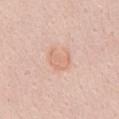Recorded during total-body skin imaging; not selected for excision or biopsy.
Measured at roughly 3.5 mm in maximum diameter.
The lesion is located on the abdomen.
The subject is a female in their mid-40s.
A roughly 15 mm field-of-view crop from a total-body skin photograph.
The lesion-visualizer software estimated a lesion area of about 7.5 mm². It also reported a mean CIELAB color near L≈69 a*≈21 b*≈30, a lesion–skin lightness drop of about 6, and a normalized lesion–skin contrast near 5. The analysis additionally found a lesion-detection confidence of about 100/100.